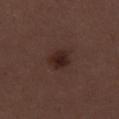{"biopsy_status": "not biopsied; imaged during a skin examination", "image": {"source": "total-body photography crop", "field_of_view_mm": 15}, "patient": {"sex": "female", "age_approx": 50}, "site": "right thigh", "lighting": "white-light", "lesion_size": {"long_diameter_mm_approx": 2.5}}